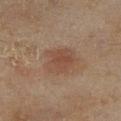lighting: cross-polarized
automated_metrics:
  eccentricity: 0.6
  border_irregularity_0_10: 3.0
  color_variation_0_10: 2.5
  peripheral_color_asymmetry: 1.0
  nevus_likeness_0_100: 45
  lesion_detection_confidence_0_100: 100
patient:
  sex: female
  age_approx: 60
site: leg
lesion_size:
  long_diameter_mm_approx: 3.5
image:
  source: total-body photography crop
  field_of_view_mm: 15